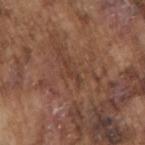image source: ~15 mm tile from a whole-body skin photo
site: the arm
size: ~3 mm (longest diameter)
lighting: white-light
patient: male, approximately 75 years of age
image-analysis metrics: a lesion area of about 2 mm², an outline eccentricity of about 0.95 (0 = round, 1 = elongated), and a shape-asymmetry score of about 0.45 (0 = symmetric); an average lesion color of about L≈39 a*≈20 b*≈27 (CIELAB), about 6 CIELAB-L* units darker than the surrounding skin, and a lesion-to-skin contrast of about 5 (normalized; higher = more distinct)